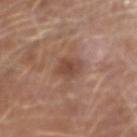workup — catalogued during a skin exam; not biopsied
patient — male, approximately 65 years of age
acquisition — ~15 mm crop, total-body skin-cancer survey
site — the arm